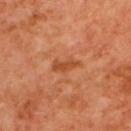The lesion was photographed on a routine skin check and not biopsied; there is no pathology result.
A female subject, aged approximately 40.
The lesion is located on the upper back.
A lesion tile, about 15 mm wide, cut from a 3D total-body photograph.